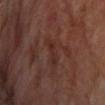workup = imaged on a skin check; not biopsied | patient = male, roughly 65 years of age | imaging modality = ~15 mm crop, total-body skin-cancer survey | tile lighting = cross-polarized | lesion size = ≈3.5 mm | body site = the chest | image-analysis metrics = border irregularity of about 4.5 on a 0–10 scale, internal color variation of about 0.5 on a 0–10 scale, and a peripheral color-asymmetry measure near 0.